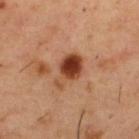| field | value |
|---|---|
| illumination | cross-polarized illumination |
| image | 15 mm crop, total-body photography |
| subject | male, in their mid-50s |
| diameter | ~4.5 mm (longest diameter) |
| body site | the upper back |
| automated lesion analysis | a mean CIELAB color near L≈42 a*≈25 b*≈33, about 15 CIELAB-L* units darker than the surrounding skin, and a normalized lesion–skin contrast near 11.5; border irregularity of about 3.5 on a 0–10 scale, a within-lesion color-variation index near 6.5/10, and radial color variation of about 2.5; an automated nevus-likeness rating near 100 out of 100 and a lesion-detection confidence of about 100/100 |